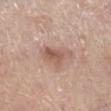No biopsy was performed on this lesion — it was imaged during a full skin examination and was not determined to be concerning.